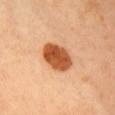Part of a total-body skin-imaging series; this lesion was reviewed on a skin check and was not flagged for biopsy. The lesion is located on the front of the torso. An algorithmic analysis of the crop reported a footprint of about 11 mm², a shape eccentricity near 0.7, and a shape-asymmetry score of about 0.1 (0 = symmetric). It also reported an average lesion color of about L≈53 a*≈29 b*≈41 (CIELAB), roughly 18 lightness units darker than nearby skin, and a lesion-to-skin contrast of about 12 (normalized; higher = more distinct). The software also gave a classifier nevus-likeness of about 100/100 and a lesion-detection confidence of about 100/100. The patient is a female approximately 35 years of age. A close-up tile cropped from a whole-body skin photograph, about 15 mm across. This is a cross-polarized tile.